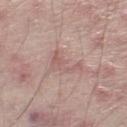Notes:
- notes: imaged on a skin check; not biopsied
- illumination: white-light
- body site: the leg
- TBP lesion metrics: a footprint of about 5 mm², an eccentricity of roughly 0.85, and a shape-asymmetry score of about 0.75 (0 = symmetric); a mean CIELAB color near L≈57 a*≈20 b*≈22, roughly 7 lightness units darker than nearby skin, and a normalized lesion–skin contrast near 5
- image: ~15 mm tile from a whole-body skin photo
- subject: male, aged around 65
- lesion size: ≈4 mm